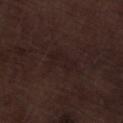Findings:
– notes: no biopsy performed (imaged during a skin exam)
– patient: male, roughly 70 years of age
– acquisition: 15 mm crop, total-body photography
– tile lighting: white-light
– location: the left lower leg